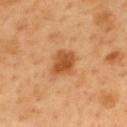Q: Was a biopsy performed?
A: catalogued during a skin exam; not biopsied
Q: Who is the patient?
A: male, approximately 50 years of age
Q: Lesion location?
A: the mid back
Q: What kind of image is this?
A: ~15 mm tile from a whole-body skin photo